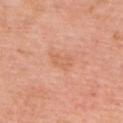Q: Is there a histopathology result?
A: catalogued during a skin exam; not biopsied
Q: Patient demographics?
A: male, aged around 75
Q: What is the anatomic site?
A: the upper back
Q: What lighting was used for the tile?
A: white-light illumination
Q: What kind of image is this?
A: ~15 mm crop, total-body skin-cancer survey